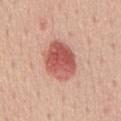Case summary:
– follow-up: imaged on a skin check; not biopsied
– imaging modality: ~15 mm crop, total-body skin-cancer survey
– anatomic site: the back
– subject: male, in their mid-50s